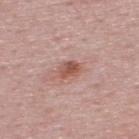Impression: The lesion was photographed on a routine skin check and not biopsied; there is no pathology result. Acquisition and patient details: The total-body-photography lesion software estimated an eccentricity of roughly 0.65 and a symmetry-axis asymmetry near 0.2. The software also gave a mean CIELAB color near L≈53 a*≈24 b*≈26 and a lesion–skin lightness drop of about 11. The software also gave a lesion-detection confidence of about 100/100. This is a white-light tile. From the upper back. The recorded lesion diameter is about 2.5 mm. A male patient aged around 50. A roughly 15 mm field-of-view crop from a total-body skin photograph.